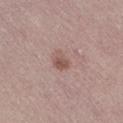Captured during whole-body skin photography for melanoma surveillance; the lesion was not biopsied. On the right thigh. Approximately 2.5 mm at its widest. A female patient, aged 38 to 42. Imaged with white-light lighting. A region of skin cropped from a whole-body photographic capture, roughly 15 mm wide.